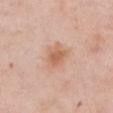Q: Was this lesion biopsied?
A: total-body-photography surveillance lesion; no biopsy
Q: What is the lesion's diameter?
A: ~4 mm (longest diameter)
Q: Automated lesion metrics?
A: an area of roughly 8.5 mm², an outline eccentricity of about 0.6 (0 = round, 1 = elongated), and a symmetry-axis asymmetry near 0.25; a lesion color around L≈63 a*≈21 b*≈33 in CIELAB, roughly 9 lightness units darker than nearby skin, and a normalized lesion–skin contrast near 7
Q: What is the imaging modality?
A: total-body-photography crop, ~15 mm field of view
Q: What is the anatomic site?
A: the chest
Q: What are the patient's age and sex?
A: female, in their mid- to late 70s